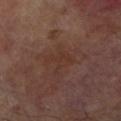| key | value |
|---|---|
| biopsy status | no biopsy performed (imaged during a skin exam) |
| patient | male, roughly 70 years of age |
| anatomic site | the right lower leg |
| image source | 15 mm crop, total-body photography |